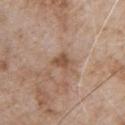The lesion was tiled from a total-body skin photograph and was not biopsied.
The lesion is on the chest.
A male subject aged around 80.
This is a white-light tile.
About 2.5 mm across.
The lesion-visualizer software estimated a shape eccentricity near 0.65 and a shape-asymmetry score of about 0.35 (0 = symmetric). The software also gave a lesion color around L≈52 a*≈18 b*≈30 in CIELAB, roughly 10 lightness units darker than nearby skin, and a normalized lesion–skin contrast near 7.5.
This image is a 15 mm lesion crop taken from a total-body photograph.